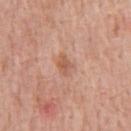This lesion was catalogued during total-body skin photography and was not selected for biopsy. The lesion is located on the chest. A 15 mm close-up tile from a total-body photography series done for melanoma screening. About 2.5 mm across. This is a white-light tile. Automated image analysis of the tile measured a classifier nevus-likeness of about 0/100 and a lesion-detection confidence of about 100/100. A male patient, in their 60s.